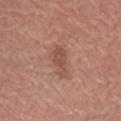* workup — total-body-photography surveillance lesion; no biopsy
* site — the left forearm
* patient — female, aged 63 to 67
* lesion size — ~4 mm (longest diameter)
* image source — 15 mm crop, total-body photography
* lighting — white-light